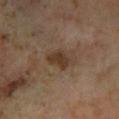{"biopsy_status": "not biopsied; imaged during a skin examination", "lighting": "cross-polarized", "automated_metrics": {"color_variation_0_10": 3.0, "peripheral_color_asymmetry": 1.0}, "site": "leg", "image": {"source": "total-body photography crop", "field_of_view_mm": 15}, "lesion_size": {"long_diameter_mm_approx": 3.5}, "patient": {"sex": "male", "age_approx": 60}}